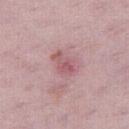Captured during whole-body skin photography for melanoma surveillance; the lesion was not biopsied. The patient is a female aged approximately 30. The lesion's longest dimension is about 3.5 mm. Imaged with white-light lighting. A region of skin cropped from a whole-body photographic capture, roughly 15 mm wide. From the right thigh. The lesion-visualizer software estimated border irregularity of about 3.5 on a 0–10 scale, internal color variation of about 4 on a 0–10 scale, and peripheral color asymmetry of about 1.5.